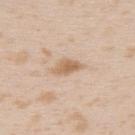Captured during whole-body skin photography for melanoma surveillance; the lesion was not biopsied. The lesion is on the upper back. A female patient, aged approximately 25. Captured under white-light illumination. About 3.5 mm across. The lesion-visualizer software estimated a normalized lesion–skin contrast near 7.5. Cropped from a whole-body photographic skin survey; the tile spans about 15 mm.